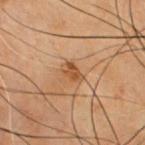Imaged during a routine full-body skin examination; the lesion was not biopsied and no histopathology is available.